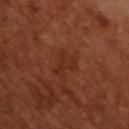| key | value |
|---|---|
| workup | catalogued during a skin exam; not biopsied |
| patient | male, roughly 65 years of age |
| imaging modality | ~15 mm tile from a whole-body skin photo |
| illumination | cross-polarized |
| diameter | ~3 mm (longest diameter) |